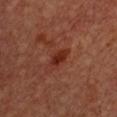<record>
  <biopsy_status>not biopsied; imaged during a skin examination</biopsy_status>
  <image>
    <source>total-body photography crop</source>
    <field_of_view_mm>15</field_of_view_mm>
  </image>
  <patient>
    <sex>female</sex>
    <age_approx>50</age_approx>
  </patient>
  <automated_metrics>
    <vs_skin_darker_L>7.0</vs_skin_darker_L>
    <vs_skin_contrast_norm>8.0</vs_skin_contrast_norm>
    <border_irregularity_0_10>3.0</border_irregularity_0_10>
    <color_variation_0_10>2.0</color_variation_0_10>
    <peripheral_color_asymmetry>0.5</peripheral_color_asymmetry>
    <nevus_likeness_0_100>25</nevus_likeness_0_100>
    <lesion_detection_confidence_0_100>100</lesion_detection_confidence_0_100>
  </automated_metrics>
  <site>chest</site>
  <lesion_size>
    <long_diameter_mm_approx>3.5</long_diameter_mm_approx>
  </lesion_size>
  <lighting>cross-polarized</lighting>
</record>